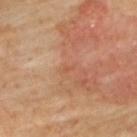No biopsy was performed on this lesion — it was imaged during a full skin examination and was not determined to be concerning.